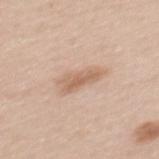A female patient aged 33 to 37. The recorded lesion diameter is about 5 mm. The lesion is located on the upper back. The tile uses white-light illumination. Automated tile analysis of the lesion measured a lesion color around L≈64 a*≈18 b*≈30 in CIELAB, a lesion–skin lightness drop of about 9, and a normalized lesion–skin contrast near 6.5. The analysis additionally found border irregularity of about 3.5 on a 0–10 scale, internal color variation of about 3 on a 0–10 scale, and radial color variation of about 1. A close-up tile cropped from a whole-body skin photograph, about 15 mm across.